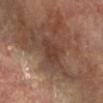This lesion was catalogued during total-body skin photography and was not selected for biopsy.
About 3 mm across.
The tile uses cross-polarized illumination.
Cropped from a total-body skin-imaging series; the visible field is about 15 mm.
The lesion is on the left lower leg.
A female patient, aged approximately 75.